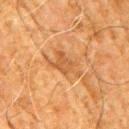Findings:
- notes: no biopsy performed (imaged during a skin exam)
- TBP lesion metrics: an eccentricity of roughly 0.8; a classifier nevus-likeness of about 0/100 and a lesion-detection confidence of about 90/100
- image source: 15 mm crop, total-body photography
- size: about 4.5 mm
- anatomic site: the arm
- subject: male, aged 78–82
- lighting: cross-polarized